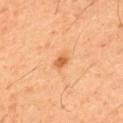follow-up = total-body-photography surveillance lesion; no biopsy | acquisition = ~15 mm tile from a whole-body skin photo | diameter = ~2 mm (longest diameter) | anatomic site = the upper back | subject = male, aged 58 to 62.